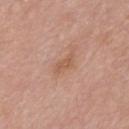Part of a total-body skin-imaging series; this lesion was reviewed on a skin check and was not flagged for biopsy.
This is a white-light tile.
A region of skin cropped from a whole-body photographic capture, roughly 15 mm wide.
An algorithmic analysis of the crop reported a footprint of about 3 mm² and an eccentricity of roughly 0.9. It also reported a lesion color around L≈56 a*≈22 b*≈32 in CIELAB and a lesion–skin lightness drop of about 7. The analysis additionally found a border-irregularity index near 4/10. The analysis additionally found an automated nevus-likeness rating near 0 out of 100 and a lesion-detection confidence of about 100/100.
On the chest.
The patient is a male aged 53 to 57.